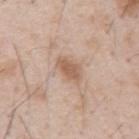{
  "biopsy_status": "not biopsied; imaged during a skin examination",
  "patient": {
    "sex": "male",
    "age_approx": 55
  },
  "image": {
    "source": "total-body photography crop",
    "field_of_view_mm": 15
  }
}